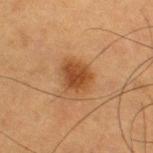Case summary:
• notes: total-body-photography surveillance lesion; no biopsy
• subject: male, about 55 years old
• location: the right thigh
• image source: ~15 mm crop, total-body skin-cancer survey